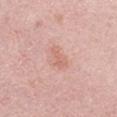On the right upper arm.
The lesion-visualizer software estimated a lesion color around L≈64 a*≈23 b*≈28 in CIELAB, roughly 7 lightness units darker than nearby skin, and a normalized border contrast of about 5.5. It also reported border irregularity of about 4 on a 0–10 scale, internal color variation of about 0.5 on a 0–10 scale, and radial color variation of about 0.
Approximately 3 mm at its widest.
A close-up tile cropped from a whole-body skin photograph, about 15 mm across.
A male patient aged approximately 25.